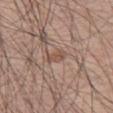Image and clinical context: Measured at roughly 2.5 mm in maximum diameter. A close-up tile cropped from a whole-body skin photograph, about 15 mm across. The lesion is on the left thigh. The subject is a male in their mid- to late 50s. Captured under white-light illumination.